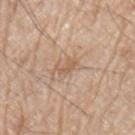Impression:
Imaged during a routine full-body skin examination; the lesion was not biopsied and no histopathology is available.
Context:
Cropped from a whole-body photographic skin survey; the tile spans about 15 mm. On the arm. The patient is a male about 70 years old.